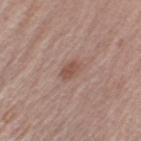Captured during whole-body skin photography for melanoma surveillance; the lesion was not biopsied. On the left upper arm. A male subject about 65 years old. A 15 mm crop from a total-body photograph taken for skin-cancer surveillance. The tile uses white-light illumination. Automated tile analysis of the lesion measured a footprint of about 3.5 mm² and an outline eccentricity of about 0.75 (0 = round, 1 = elongated). It also reported an average lesion color of about L≈51 a*≈20 b*≈25 (CIELAB), about 9 CIELAB-L* units darker than the surrounding skin, and a normalized lesion–skin contrast near 6.5.